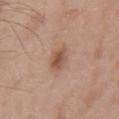This is a white-light tile. A male patient, aged around 65. The lesion is on the chest. Approximately 4 mm at its widest. A 15 mm close-up tile from a total-body photography series done for melanoma screening. Automated image analysis of the tile measured a footprint of about 6 mm², an eccentricity of roughly 0.85, and a symmetry-axis asymmetry near 0.2. It also reported an average lesion color of about L≈53 a*≈20 b*≈29 (CIELAB) and a normalized border contrast of about 7. The analysis additionally found a classifier nevus-likeness of about 80/100.